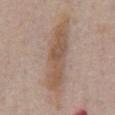The lesion was tiled from a total-body skin photograph and was not biopsied. Imaged with white-light lighting. Measured at roughly 9.5 mm in maximum diameter. A male subject aged around 55. A region of skin cropped from a whole-body photographic capture, roughly 15 mm wide. The total-body-photography lesion software estimated a lesion area of about 22 mm², an eccentricity of roughly 0.95, and a shape-asymmetry score of about 0.25 (0 = symmetric). And it measured a border-irregularity index near 4/10 and a within-lesion color-variation index near 3.5/10. It also reported lesion-presence confidence of about 80/100. On the abdomen.